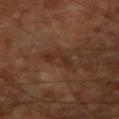Clinical impression:
Part of a total-body skin-imaging series; this lesion was reviewed on a skin check and was not flagged for biopsy.
Background:
Located on the right upper arm. Imaged with cross-polarized lighting. Longest diameter approximately 3.5 mm. The patient is approximately 65 years of age. A close-up tile cropped from a whole-body skin photograph, about 15 mm across.